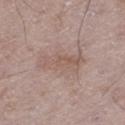Part of a total-body skin-imaging series; this lesion was reviewed on a skin check and was not flagged for biopsy. A male patient aged 48 to 52. Located on the left thigh. Cropped from a whole-body photographic skin survey; the tile spans about 15 mm.